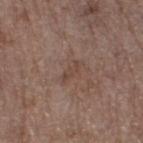workup: imaged on a skin check; not biopsied | size: ≈3 mm | lighting: white-light illumination | TBP lesion metrics: an outline eccentricity of about 0.95 (0 = round, 1 = elongated); an average lesion color of about L≈44 a*≈17 b*≈24 (CIELAB); border irregularity of about 5 on a 0–10 scale, internal color variation of about 0 on a 0–10 scale, and a peripheral color-asymmetry measure near 0; an automated nevus-likeness rating near 0 out of 100 and a lesion-detection confidence of about 100/100 | anatomic site: the right thigh | imaging modality: 15 mm crop, total-body photography | subject: female, aged 63–67.